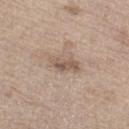notes: imaged on a skin check; not biopsied.